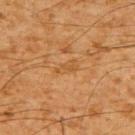Part of a total-body skin-imaging series; this lesion was reviewed on a skin check and was not flagged for biopsy. The tile uses cross-polarized illumination. The lesion is located on the upper back. This image is a 15 mm lesion crop taken from a total-body photograph. About 3 mm across. An algorithmic analysis of the crop reported an average lesion color of about L≈44 a*≈19 b*≈37 (CIELAB) and a normalized border contrast of about 4.5. It also reported an automated nevus-likeness rating near 0 out of 100. A male subject about 60 years old.